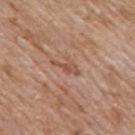Case summary:
• image — 15 mm crop, total-body photography
• subject — male, aged 58–62
• body site — the mid back